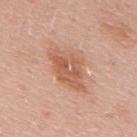  biopsy_status: not biopsied; imaged during a skin examination
  lesion_size:
    long_diameter_mm_approx: 6.0
  site: mid back
  image:
    source: total-body photography crop
    field_of_view_mm: 15
  lighting: white-light
  patient:
    sex: male
    age_approx: 55